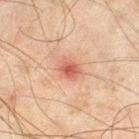  biopsy_status: not biopsied; imaged during a skin examination
  patient:
    sex: male
    age_approx: 45
  image:
    source: total-body photography crop
    field_of_view_mm: 15
  site: leg
  lesion_size:
    long_diameter_mm_approx: 2.5